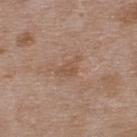<case>
  <biopsy_status>not biopsied; imaged during a skin examination</biopsy_status>
  <image>
    <source>total-body photography crop</source>
    <field_of_view_mm>15</field_of_view_mm>
  </image>
  <site>upper back</site>
  <patient>
    <sex>male</sex>
    <age_approx>50</age_approx>
  </patient>
</case>